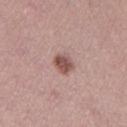Clinical impression:
Imaged during a routine full-body skin examination; the lesion was not biopsied and no histopathology is available.
Acquisition and patient details:
A female patient approximately 40 years of age. This image is a 15 mm lesion crop taken from a total-body photograph. The lesion is on the left thigh.